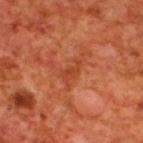Recorded during total-body skin imaging; not selected for excision or biopsy. The subject is a male about 70 years old. Measured at roughly 2.5 mm in maximum diameter. The lesion is located on the upper back. A roughly 15 mm field-of-view crop from a total-body skin photograph.